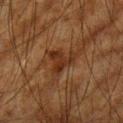biopsy status: catalogued during a skin exam; not biopsied | illumination: cross-polarized illumination | anatomic site: the left upper arm | image: ~15 mm crop, total-body skin-cancer survey | subject: male, approximately 65 years of age | size: ~4.5 mm (longest diameter) | automated metrics: an eccentricity of roughly 0.65 and a symmetry-axis asymmetry near 0.6; a mean CIELAB color near L≈25 a*≈18 b*≈26, about 7 CIELAB-L* units darker than the surrounding skin, and a lesion-to-skin contrast of about 8 (normalized; higher = more distinct); a nevus-likeness score of about 0/100 and a detector confidence of about 80 out of 100 that the crop contains a lesion.